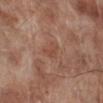follow-up: imaged on a skin check; not biopsied | diameter: ≈2.5 mm | automated metrics: an area of roughly 3.5 mm², a shape eccentricity near 0.75, and a symmetry-axis asymmetry near 0.35; an average lesion color of about L≈47 a*≈23 b*≈29 (CIELAB), roughly 6 lightness units darker than nearby skin, and a normalized border contrast of about 5 | image: total-body-photography crop, ~15 mm field of view | site: the right lower leg | patient: male, roughly 70 years of age | illumination: white-light.